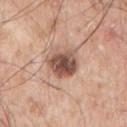This lesion was catalogued during total-body skin photography and was not selected for biopsy. Cropped from a whole-body photographic skin survey; the tile spans about 15 mm. A male subject, roughly 70 years of age. The lesion is located on the left arm. Captured under white-light illumination. Measured at roughly 4 mm in maximum diameter. Automated image analysis of the tile measured internal color variation of about 6 on a 0–10 scale and radial color variation of about 1.5. The software also gave a classifier nevus-likeness of about 60/100 and a lesion-detection confidence of about 100/100.